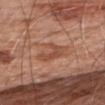Q: Is there a histopathology result?
A: catalogued during a skin exam; not biopsied
Q: Lesion size?
A: about 3.5 mm
Q: What lighting was used for the tile?
A: white-light
Q: Patient demographics?
A: male, in their 80s
Q: What kind of image is this?
A: ~15 mm crop, total-body skin-cancer survey
Q: Lesion location?
A: the back